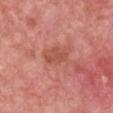biopsy status = catalogued during a skin exam; not biopsied | subject = female, in their 40s | TBP lesion metrics = a shape eccentricity near 0.8 and two-axis asymmetry of about 0.2; a lesion color around L≈52 a*≈29 b*≈30 in CIELAB, roughly 8 lightness units darker than nearby skin, and a normalized border contrast of about 5.5 | lesion diameter = ≈3.5 mm | body site = the chest | image = ~15 mm tile from a whole-body skin photo | lighting = white-light.